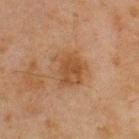Q: Was this lesion biopsied?
A: total-body-photography surveillance lesion; no biopsy
Q: What is the imaging modality?
A: ~15 mm crop, total-body skin-cancer survey
Q: What are the patient's age and sex?
A: male, approximately 45 years of age
Q: Illumination type?
A: cross-polarized illumination
Q: What is the lesion's diameter?
A: ≈4 mm
Q: What is the anatomic site?
A: the upper back